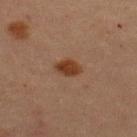workup: total-body-photography surveillance lesion; no biopsy
lighting: cross-polarized
subject: male, in their 60s
image: ~15 mm tile from a whole-body skin photo
automated metrics: an area of roughly 5 mm² and two-axis asymmetry of about 0.2; a lesion color around L≈31 a*≈18 b*≈27 in CIELAB, a lesion–skin lightness drop of about 9, and a lesion-to-skin contrast of about 10 (normalized; higher = more distinct); an automated nevus-likeness rating near 100 out of 100 and lesion-presence confidence of about 100/100
site: the back